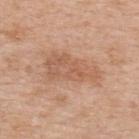{
  "biopsy_status": "not biopsied; imaged during a skin examination",
  "patient": {
    "sex": "male",
    "age_approx": 70
  },
  "lighting": "white-light",
  "site": "upper back",
  "lesion_size": {
    "long_diameter_mm_approx": 6.5
  },
  "automated_metrics": {
    "area_mm2_approx": 17.0,
    "eccentricity": 0.9,
    "shape_asymmetry": 0.4,
    "cielab_L": 59,
    "cielab_a": 21,
    "cielab_b": 32,
    "vs_skin_darker_L": 8.0,
    "vs_skin_contrast_norm": 5.5,
    "lesion_detection_confidence_0_100": 100
  },
  "image": {
    "source": "total-body photography crop",
    "field_of_view_mm": 15
  }
}